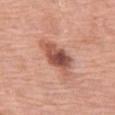image: ~15 mm tile from a whole-body skin photo
subject: female, in their mid-60s
diameter: ~5.5 mm (longest diameter)
lighting: white-light illumination
anatomic site: the left thigh
automated lesion analysis: an area of roughly 11 mm² and an outline eccentricity of about 0.85 (0 = round, 1 = elongated); a mean CIELAB color near L≈53 a*≈25 b*≈29 and about 14 CIELAB-L* units darker than the surrounding skin; a border-irregularity rating of about 3.5/10 and a peripheral color-asymmetry measure near 2.5; a nevus-likeness score of about 45/100